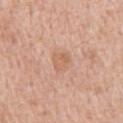No biopsy was performed on this lesion — it was imaged during a full skin examination and was not determined to be concerning. The lesion is on the chest. Cropped from a total-body skin-imaging series; the visible field is about 15 mm. An algorithmic analysis of the crop reported an area of roughly 4.5 mm², a shape eccentricity near 0.7, and two-axis asymmetry of about 0.3. It also reported about 7 CIELAB-L* units darker than the surrounding skin and a lesion-to-skin contrast of about 5 (normalized; higher = more distinct). It also reported peripheral color asymmetry of about 1. Imaged with white-light lighting. A male patient, approximately 60 years of age.